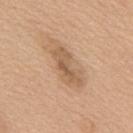Q: Was a biopsy performed?
A: catalogued during a skin exam; not biopsied
Q: Where on the body is the lesion?
A: the upper back
Q: Automated lesion metrics?
A: an eccentricity of roughly 0.9 and a shape-asymmetry score of about 0.3 (0 = symmetric); a lesion color around L≈58 a*≈18 b*≈33 in CIELAB, roughly 10 lightness units darker than nearby skin, and a lesion-to-skin contrast of about 6.5 (normalized; higher = more distinct); a classifier nevus-likeness of about 25/100
Q: How was this image acquired?
A: ~15 mm crop, total-body skin-cancer survey
Q: Lesion size?
A: about 5.5 mm
Q: Illumination type?
A: white-light illumination
Q: Who is the patient?
A: male, aged 58–62